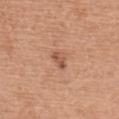{
  "biopsy_status": "not biopsied; imaged during a skin examination",
  "automated_metrics": {
    "border_irregularity_0_10": 4.0,
    "color_variation_0_10": 2.0,
    "peripheral_color_asymmetry": 0.5
  },
  "site": "upper back",
  "lesion_size": {
    "long_diameter_mm_approx": 2.5
  },
  "patient": {
    "sex": "female",
    "age_approx": 60
  },
  "lighting": "white-light",
  "image": {
    "source": "total-body photography crop",
    "field_of_view_mm": 15
  }
}